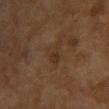Assessment: Captured during whole-body skin photography for melanoma surveillance; the lesion was not biopsied. Acquisition and patient details: About 3 mm across. On the chest. The tile uses cross-polarized illumination. An algorithmic analysis of the crop reported an area of roughly 4.5 mm², an eccentricity of roughly 0.65, and two-axis asymmetry of about 0.4. It also reported a border-irregularity rating of about 4/10, a color-variation rating of about 2/10, and radial color variation of about 0.5. The software also gave a detector confidence of about 100 out of 100 that the crop contains a lesion. Cropped from a total-body skin-imaging series; the visible field is about 15 mm. A female subject, aged 58 to 62.